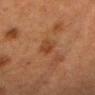Q: Was this lesion biopsied?
A: catalogued during a skin exam; not biopsied
Q: What are the patient's age and sex?
A: female, roughly 40 years of age
Q: What is the lesion's diameter?
A: about 2.5 mm
Q: What is the imaging modality?
A: 15 mm crop, total-body photography
Q: What did automated image analysis measure?
A: an eccentricity of roughly 0.75 and two-axis asymmetry of about 0.25; lesion-presence confidence of about 100/100
Q: Where on the body is the lesion?
A: the head or neck